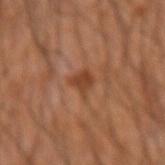Clinical impression:
The lesion was tiled from a total-body skin photograph and was not biopsied.
Acquisition and patient details:
Imaged with cross-polarized lighting. Measured at roughly 2.5 mm in maximum diameter. A male subject aged 43 to 47. On the right forearm. This image is a 15 mm lesion crop taken from a total-body photograph. Automated image analysis of the tile measured border irregularity of about 4 on a 0–10 scale, internal color variation of about 2 on a 0–10 scale, and peripheral color asymmetry of about 1. The analysis additionally found an automated nevus-likeness rating near 40 out of 100.